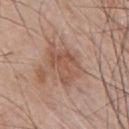Captured during whole-body skin photography for melanoma surveillance; the lesion was not biopsied. Located on the chest. A male patient, about 55 years old. A region of skin cropped from a whole-body photographic capture, roughly 15 mm wide.